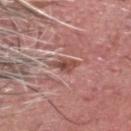Q: Is there a histopathology result?
A: no biopsy performed (imaged during a skin exam)
Q: What is the anatomic site?
A: the head or neck
Q: How large is the lesion?
A: ~3 mm (longest diameter)
Q: Automated lesion metrics?
A: an area of roughly 3.5 mm² and a shape-asymmetry score of about 0.35 (0 = symmetric); a lesion–skin lightness drop of about 10; a border-irregularity index near 3.5/10, internal color variation of about 3 on a 0–10 scale, and radial color variation of about 1; an automated nevus-likeness rating near 0 out of 100
Q: What kind of image is this?
A: 15 mm crop, total-body photography
Q: Who is the patient?
A: male, aged around 75
Q: What lighting was used for the tile?
A: white-light illumination